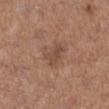biopsy status: no biopsy performed (imaged during a skin exam); lesion diameter: ≈3 mm; acquisition: ~15 mm tile from a whole-body skin photo; site: the right lower leg; patient: female, aged around 65.